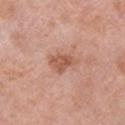Case summary:
* workup: catalogued during a skin exam; not biopsied
* automated metrics: a border-irregularity index near 3.5/10, a color-variation rating of about 3/10, and a peripheral color-asymmetry measure near 1; a classifier nevus-likeness of about 5/100 and lesion-presence confidence of about 100/100
* image: 15 mm crop, total-body photography
* lesion size: ≈3.5 mm
* subject: male, approximately 55 years of age
* location: the left upper arm